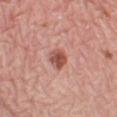Clinical summary: Captured under white-light illumination. A 15 mm close-up tile from a total-body photography series done for melanoma screening. Measured at roughly 2.5 mm in maximum diameter. The lesion is located on the left thigh. A female subject, approximately 65 years of age.